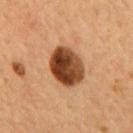Q: Lesion location?
A: the back
Q: What kind of image is this?
A: 15 mm crop, total-body photography
Q: Lesion size?
A: about 5 mm
Q: Who is the patient?
A: male, aged approximately 55